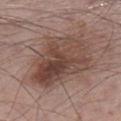{
  "biopsy_status": "not biopsied; imaged during a skin examination",
  "patient": {
    "sex": "male",
    "age_approx": 55
  },
  "lesion_size": {
    "long_diameter_mm_approx": 8.5
  },
  "image": {
    "source": "total-body photography crop",
    "field_of_view_mm": 15
  },
  "site": "left lower leg",
  "lighting": "white-light"
}